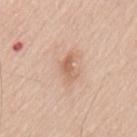Imaged during a routine full-body skin examination; the lesion was not biopsied and no histopathology is available. A 15 mm crop from a total-body photograph taken for skin-cancer surveillance. A male patient aged approximately 65. On the mid back.